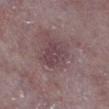{"biopsy_status": "not biopsied; imaged during a skin examination"}